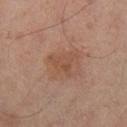Context: A male subject, in their 50s. A 15 mm close-up extracted from a 3D total-body photography capture. On the left leg.